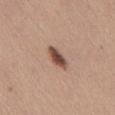• patient — female, aged around 55
• image — total-body-photography crop, ~15 mm field of view
• anatomic site — the front of the torso
• size — ≈3.5 mm